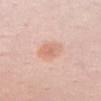follow-up: imaged on a skin check; not biopsied
image source: ~15 mm crop, total-body skin-cancer survey
subject: female, aged approximately 45
diameter: about 3.5 mm
site: the chest
TBP lesion metrics: a footprint of about 7.5 mm², an outline eccentricity of about 0.7 (0 = round, 1 = elongated), and a shape-asymmetry score of about 0.2 (0 = symmetric); an average lesion color of about L≈69 a*≈22 b*≈30 (CIELAB) and a lesion–skin lightness drop of about 8; a nevus-likeness score of about 85/100 and a detector confidence of about 100 out of 100 that the crop contains a lesion
lighting: white-light